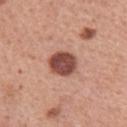Clinical impression: Captured during whole-body skin photography for melanoma surveillance; the lesion was not biopsied. Acquisition and patient details: The patient is a female aged approximately 60. Approximately 3.5 mm at its widest. A 15 mm close-up extracted from a 3D total-body photography capture. Located on the left upper arm. This is a white-light tile.